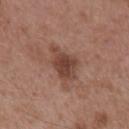Q: Was this lesion biopsied?
A: total-body-photography surveillance lesion; no biopsy
Q: Who is the patient?
A: male, about 55 years old
Q: How was the tile lit?
A: white-light illumination
Q: What kind of image is this?
A: ~15 mm tile from a whole-body skin photo
Q: Lesion location?
A: the mid back
Q: Lesion size?
A: ~4.5 mm (longest diameter)
Q: What did automated image analysis measure?
A: a shape eccentricity near 0.8 and a symmetry-axis asymmetry near 0.3; a mean CIELAB color near L≈43 a*≈21 b*≈26, a lesion–skin lightness drop of about 11, and a normalized lesion–skin contrast near 8.5; a classifier nevus-likeness of about 60/100 and a lesion-detection confidence of about 100/100